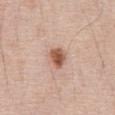<record>
  <biopsy_status>not biopsied; imaged during a skin examination</biopsy_status>
  <lighting>white-light</lighting>
  <automated_metrics>
    <cielab_L>56</cielab_L>
    <cielab_a>22</cielab_a>
    <cielab_b>31</cielab_b>
    <vs_skin_darker_L>15.0</vs_skin_darker_L>
    <vs_skin_contrast_norm>10.0</vs_skin_contrast_norm>
    <border_irregularity_0_10>1.5</border_irregularity_0_10>
    <color_variation_0_10>3.5</color_variation_0_10>
    <peripheral_color_asymmetry>1.0</peripheral_color_asymmetry>
    <nevus_likeness_0_100>100</nevus_likeness_0_100>
    <lesion_detection_confidence_0_100>100</lesion_detection_confidence_0_100>
  </automated_metrics>
  <lesion_size>
    <long_diameter_mm_approx>2.5</long_diameter_mm_approx>
  </lesion_size>
  <site>front of the torso</site>
  <patient>
    <sex>male</sex>
    <age_approx>70</age_approx>
  </patient>
  <image>
    <source>total-body photography crop</source>
    <field_of_view_mm>15</field_of_view_mm>
  </image>
</record>